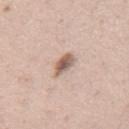| feature | finding |
|---|---|
| biopsy status | catalogued during a skin exam; not biopsied |
| patient | male, in their 40s |
| image source | ~15 mm tile from a whole-body skin photo |
| site | the abdomen |
| lesion size | about 3 mm |